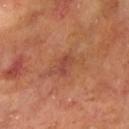The lesion was tiled from a total-body skin photograph and was not biopsied.
The subject is a female aged approximately 75.
On the right upper arm.
A close-up tile cropped from a whole-body skin photograph, about 15 mm across.